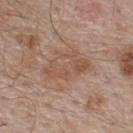Q: Is there a histopathology result?
A: imaged on a skin check; not biopsied
Q: Automated lesion metrics?
A: a lesion area of about 14 mm², an outline eccentricity of about 0.8 (0 = round, 1 = elongated), and two-axis asymmetry of about 0.35; a lesion-to-skin contrast of about 5.5 (normalized; higher = more distinct); border irregularity of about 4 on a 0–10 scale, internal color variation of about 4 on a 0–10 scale, and a peripheral color-asymmetry measure near 1.5
Q: Patient demographics?
A: male, aged 53 to 57
Q: How was this image acquired?
A: 15 mm crop, total-body photography
Q: Where on the body is the lesion?
A: the upper back